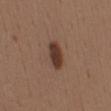No biopsy was performed on this lesion — it was imaged during a full skin examination and was not determined to be concerning. A male subject aged 38–42. From the mid back. Captured under white-light illumination. This image is a 15 mm lesion crop taken from a total-body photograph. Longest diameter approximately 4 mm.